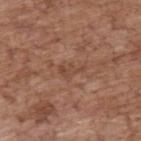Captured during whole-body skin photography for melanoma surveillance; the lesion was not biopsied.
Approximately 3 mm at its widest.
A 15 mm close-up tile from a total-body photography series done for melanoma screening.
The lesion is on the upper back.
A male patient, aged 68 to 72.
Automated tile analysis of the lesion measured border irregularity of about 7 on a 0–10 scale and a within-lesion color-variation index near 0/10.